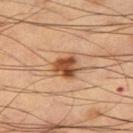This lesion was catalogued during total-body skin photography and was not selected for biopsy.
A roughly 15 mm field-of-view crop from a total-body skin photograph.
A male patient, aged 58 to 62.
The tile uses cross-polarized illumination.
From the right thigh.
The recorded lesion diameter is about 3 mm.
The total-body-photography lesion software estimated an area of roughly 7 mm², an outline eccentricity of about 0.4 (0 = round, 1 = elongated), and a shape-asymmetry score of about 0.25 (0 = symmetric). The analysis additionally found a mean CIELAB color near L≈49 a*≈24 b*≈36 and about 16 CIELAB-L* units darker than the surrounding skin. And it measured a color-variation rating of about 6.5/10 and peripheral color asymmetry of about 2.